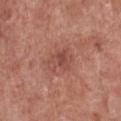<record>
<biopsy_status>not biopsied; imaged during a skin examination</biopsy_status>
<image>
  <source>total-body photography crop</source>
  <field_of_view_mm>15</field_of_view_mm>
</image>
<patient>
  <sex>female</sex>
  <age_approx>60</age_approx>
</patient>
<lighting>white-light</lighting>
<site>front of the torso</site>
<lesion_size>
  <long_diameter_mm_approx>3.5</long_diameter_mm_approx>
</lesion_size>
<automated_metrics>
  <area_mm2_approx>7.0</area_mm2_approx>
  <eccentricity>0.75</eccentricity>
  <shape_asymmetry>0.25</shape_asymmetry>
  <cielab_L>48</cielab_L>
  <cielab_a>26</cielab_a>
  <cielab_b>26</cielab_b>
  <vs_skin_contrast_norm>5.5</vs_skin_contrast_norm>
  <nevus_likeness_0_100>0</nevus_likeness_0_100>
</automated_metrics>
</record>